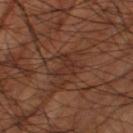Case summary:
- workup — total-body-photography surveillance lesion; no biopsy